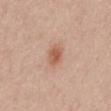A lesion tile, about 15 mm wide, cut from a 3D total-body photograph.
The lesion is on the mid back.
A female subject, aged 38–42.